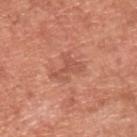The lesion was photographed on a routine skin check and not biopsied; there is no pathology result.